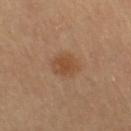{
  "biopsy_status": "not biopsied; imaged during a skin examination",
  "patient": {
    "sex": "female",
    "age_approx": 60
  },
  "image": {
    "source": "total-body photography crop",
    "field_of_view_mm": 15
  },
  "site": "leg"
}